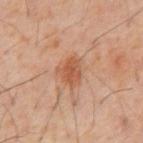Recorded during total-body skin imaging; not selected for excision or biopsy. A roughly 15 mm field-of-view crop from a total-body skin photograph. Located on the abdomen. The lesion's longest dimension is about 3.5 mm. Imaged with cross-polarized lighting. An algorithmic analysis of the crop reported an eccentricity of roughly 0.65 and two-axis asymmetry of about 0.25. The analysis additionally found a mean CIELAB color near L≈50 a*≈23 b*≈32 and about 9 CIELAB-L* units darker than the surrounding skin. The analysis additionally found a detector confidence of about 100 out of 100 that the crop contains a lesion. The patient is a male aged 58–62.